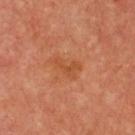Part of a total-body skin-imaging series; this lesion was reviewed on a skin check and was not flagged for biopsy. A 15 mm close-up extracted from a 3D total-body photography capture. The subject is a female roughly 50 years of age. The recorded lesion diameter is about 3.5 mm. The lesion is on the upper back.